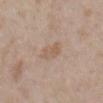Case summary:
* workup — catalogued during a skin exam; not biopsied
* image-analysis metrics — a lesion area of about 5 mm², an outline eccentricity of about 0.75 (0 = round, 1 = elongated), and a symmetry-axis asymmetry near 0.3; an average lesion color of about L≈58 a*≈15 b*≈28 (CIELAB) and a normalized lesion–skin contrast near 5; a nevus-likeness score of about 0/100 and lesion-presence confidence of about 100/100
* diameter — ≈3 mm
* subject — female, approximately 30 years of age
* image source — total-body-photography crop, ~15 mm field of view
* body site — the mid back
* tile lighting — white-light